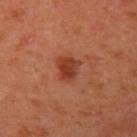workup: no biopsy performed (imaged during a skin exam); image source: 15 mm crop, total-body photography; lighting: cross-polarized illumination; lesion size: ≈2.5 mm; subject: female, roughly 40 years of age; body site: the left upper arm.